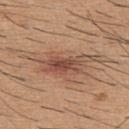No biopsy was performed on this lesion — it was imaged during a full skin examination and was not determined to be concerning.
The patient is a male aged around 60.
The lesion is located on the upper back.
A region of skin cropped from a whole-body photographic capture, roughly 15 mm wide.